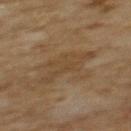follow-up — imaged on a skin check; not biopsied | lesion diameter — about 5.5 mm | image-analysis metrics — a mean CIELAB color near L≈38 a*≈13 b*≈28, roughly 5 lightness units darker than nearby skin, and a lesion-to-skin contrast of about 4.5 (normalized; higher = more distinct); an automated nevus-likeness rating near 0 out of 100 and lesion-presence confidence of about 80/100 | patient — female, aged 58 to 62 | image source — ~15 mm crop, total-body skin-cancer survey | body site — the back.